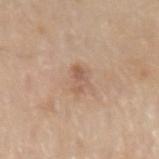Q: Is there a histopathology result?
A: catalogued during a skin exam; not biopsied
Q: What are the patient's age and sex?
A: male, about 80 years old
Q: What is the anatomic site?
A: the right arm
Q: Illumination type?
A: white-light
Q: Lesion size?
A: about 3 mm
Q: What did automated image analysis measure?
A: a lesion color around L≈58 a*≈18 b*≈30 in CIELAB, about 8 CIELAB-L* units darker than the surrounding skin, and a lesion-to-skin contrast of about 5.5 (normalized; higher = more distinct); a classifier nevus-likeness of about 0/100
Q: How was this image acquired?
A: ~15 mm tile from a whole-body skin photo